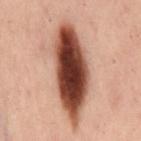Impression:
Captured during whole-body skin photography for melanoma surveillance; the lesion was not biopsied.
Clinical summary:
Automated image analysis of the tile measured an area of roughly 34 mm² and an eccentricity of roughly 0.95. It also reported an average lesion color of about L≈37 a*≈21 b*≈25 (CIELAB) and a lesion-to-skin contrast of about 16 (normalized; higher = more distinct). This is a cross-polarized tile. Approximately 11 mm at its widest. A female subject, about 55 years old. From the mid back. A close-up tile cropped from a whole-body skin photograph, about 15 mm across.